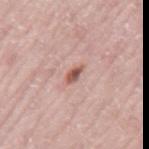The lesion was tiled from a total-body skin photograph and was not biopsied. Longest diameter approximately 2.5 mm. Automated image analysis of the tile measured a footprint of about 2.5 mm² and a shape-asymmetry score of about 0.25 (0 = symmetric). The analysis additionally found about 14 CIELAB-L* units darker than the surrounding skin and a normalized lesion–skin contrast near 9. A male patient about 75 years old. Captured under white-light illumination. A close-up tile cropped from a whole-body skin photograph, about 15 mm across. Located on the mid back.